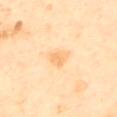The lesion was photographed on a routine skin check and not biopsied; there is no pathology result.
On the mid back.
The lesion's longest dimension is about 2.5 mm.
This is a cross-polarized tile.
Cropped from a total-body skin-imaging series; the visible field is about 15 mm.
A male subject about 65 years old.
An algorithmic analysis of the crop reported an average lesion color of about L≈80 a*≈21 b*≈45 (CIELAB) and a lesion-to-skin contrast of about 5.5 (normalized; higher = more distinct).